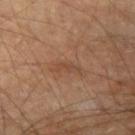biopsy status: catalogued during a skin exam; not biopsied
subject: male, about 65 years old
diameter: about 3 mm
image source: 15 mm crop, total-body photography
location: the right upper arm
image-analysis metrics: a shape eccentricity near 0.9; an average lesion color of about L≈43 a*≈19 b*≈29 (CIELAB), a lesion–skin lightness drop of about 6, and a lesion-to-skin contrast of about 5 (normalized; higher = more distinct); a border-irregularity index near 4.5/10, a color-variation rating of about 0/10, and peripheral color asymmetry of about 0; an automated nevus-likeness rating near 5 out of 100 and a lesion-detection confidence of about 100/100